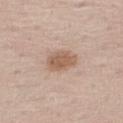Q: Was this lesion biopsied?
A: no biopsy performed (imaged during a skin exam)
Q: What is the lesion's diameter?
A: ≈3.5 mm
Q: What did automated image analysis measure?
A: an area of roughly 7 mm² and a shape eccentricity near 0.75; a lesion-to-skin contrast of about 8 (normalized; higher = more distinct)
Q: Where on the body is the lesion?
A: the leg
Q: Patient demographics?
A: male, about 65 years old
Q: What is the imaging modality?
A: ~15 mm crop, total-body skin-cancer survey
Q: Illumination type?
A: white-light